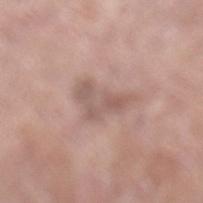Q: What is the anatomic site?
A: the left lower leg
Q: What is the lesion's diameter?
A: ~5 mm (longest diameter)
Q: How was this image acquired?
A: 15 mm crop, total-body photography
Q: What are the patient's age and sex?
A: male, aged approximately 80